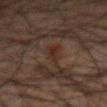Imaged during a routine full-body skin examination; the lesion was not biopsied and no histopathology is available. Imaged with cross-polarized lighting. About 3 mm across. The lesion is on the arm. The subject is a male aged around 50. A 15 mm close-up tile from a total-body photography series done for melanoma screening.